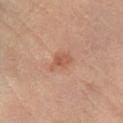biopsy_status: not biopsied; imaged during a skin examination
lighting: cross-polarized
patient:
  sex: female
  age_approx: 65
lesion_size:
  long_diameter_mm_approx: 3.0
automated_metrics:
  area_mm2_approx: 5.0
  cielab_L: 54
  cielab_a: 22
  cielab_b: 31
  vs_skin_darker_L: 8.0
  vs_skin_contrast_norm: 6.0
  peripheral_color_asymmetry: 1.0
site: left leg
image:
  source: total-body photography crop
  field_of_view_mm: 15